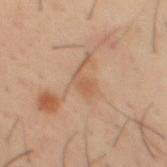Impression:
The lesion was photographed on a routine skin check and not biopsied; there is no pathology result.
Background:
A 15 mm close-up extracted from a 3D total-body photography capture. Captured under cross-polarized illumination. A male patient in their 40s. The lesion's longest dimension is about 3 mm. The lesion is on the mid back.